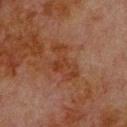Clinical impression:
Captured during whole-body skin photography for melanoma surveillance; the lesion was not biopsied.
Context:
Measured at roughly 5 mm in maximum diameter. A male subject, aged 78 to 82. The lesion is located on the upper back. Cropped from a whole-body photographic skin survey; the tile spans about 15 mm.